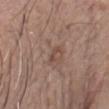biopsy status=catalogued during a skin exam; not biopsied
patient=male, aged around 60
site=the head or neck
image source=15 mm crop, total-body photography
size=~2.5 mm (longest diameter)